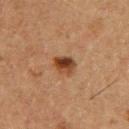{"biopsy_status": "not biopsied; imaged during a skin examination", "image": {"source": "total-body photography crop", "field_of_view_mm": 15}, "patient": {"sex": "male", "age_approx": 60}}